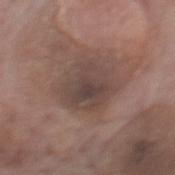| field | value |
|---|---|
| follow-up | no biopsy performed (imaged during a skin exam) |
| body site | the mid back |
| lesion size | ≈4.5 mm |
| illumination | white-light illumination |
| automated metrics | roughly 8 lightness units darker than nearby skin and a normalized border contrast of about 7 |
| subject | male, aged 73–77 |
| image | ~15 mm tile from a whole-body skin photo |